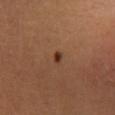Impression: Part of a total-body skin-imaging series; this lesion was reviewed on a skin check and was not flagged for biopsy. Context: A female patient in their mid-30s. A region of skin cropped from a whole-body photographic capture, roughly 15 mm wide. On the right lower leg. The tile uses cross-polarized illumination. Approximately 1.5 mm at its widest.